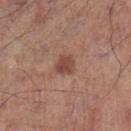| key | value |
|---|---|
| location | the leg |
| acquisition | total-body-photography crop, ~15 mm field of view |
| illumination | white-light illumination |
| patient | male, aged 68 to 72 |
| size | about 2.5 mm |